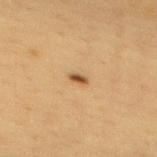subject: female, aged approximately 40 | image source: ~15 mm tile from a whole-body skin photo | size: ~2 mm (longest diameter) | body site: the mid back | illumination: cross-polarized illumination.